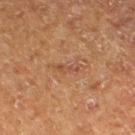Impression: The lesion was photographed on a routine skin check and not biopsied; there is no pathology result. Image and clinical context: A 15 mm crop from a total-body photograph taken for skin-cancer surveillance. The tile uses cross-polarized illumination. A male patient aged around 75. Automated tile analysis of the lesion measured a lesion color around L≈39 a*≈19 b*≈27 in CIELAB and a normalized border contrast of about 5. It also reported a color-variation rating of about 0/10 and peripheral color asymmetry of about 0. The lesion is on the left lower leg.